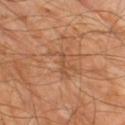| key | value |
|---|---|
| workup | catalogued during a skin exam; not biopsied |
| subject | male, aged 63 to 67 |
| tile lighting | cross-polarized |
| image | ~15 mm crop, total-body skin-cancer survey |
| image-analysis metrics | a lesion area of about 2 mm²; a nevus-likeness score of about 0/100 and a lesion-detection confidence of about 80/100 |